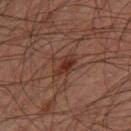The lesion was photographed on a routine skin check and not biopsied; there is no pathology result. The lesion is on the left thigh. Imaged with cross-polarized lighting. The lesion's longest dimension is about 3 mm. A male subject, in their 60s. A 15 mm close-up tile from a total-body photography series done for melanoma screening.